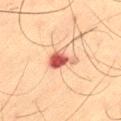Findings:
- follow-up · imaged on a skin check; not biopsied
- site · the left thigh
- imaging modality · 15 mm crop, total-body photography
- lesion size · ~3.5 mm (longest diameter)
- tile lighting · cross-polarized
- patient · male, about 65 years old
- TBP lesion metrics · an average lesion color of about L≈49 a*≈27 b*≈28 (CIELAB) and about 16 CIELAB-L* units darker than the surrounding skin; a border-irregularity rating of about 4.5/10 and internal color variation of about 4 on a 0–10 scale